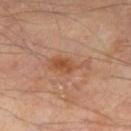notes=catalogued during a skin exam; not biopsied
body site=the right thigh
image source=~15 mm crop, total-body skin-cancer survey
illumination=cross-polarized
patient=male, approximately 65 years of age
size=about 4.5 mm